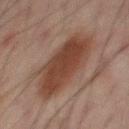notes — imaged on a skin check; not biopsied
imaging modality — ~15 mm crop, total-body skin-cancer survey
lesion size — ~8 mm (longest diameter)
patient — male, in their mid-60s
site — the mid back
automated metrics — a lesion area of about 39 mm² and an outline eccentricity of about 0.6 (0 = round, 1 = elongated); a lesion color around L≈34 a*≈15 b*≈21 in CIELAB and a lesion-to-skin contrast of about 8 (normalized; higher = more distinct); a lesion-detection confidence of about 100/100